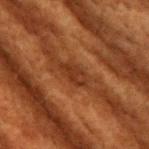Q: Is there a histopathology result?
A: total-body-photography surveillance lesion; no biopsy